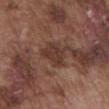biopsy_status: not biopsied; imaged during a skin examination
image:
  source: total-body photography crop
  field_of_view_mm: 15
automated_metrics:
  area_mm2_approx: 7.0
  eccentricity: 0.55
  shape_asymmetry: 0.3
  border_irregularity_0_10: 3.0
  color_variation_0_10: 2.0
  peripheral_color_asymmetry: 0.5
site: abdomen
patient:
  sex: male
  age_approx: 75
lesion_size:
  long_diameter_mm_approx: 3.5